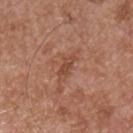Notes:
* follow-up — imaged on a skin check; not biopsied
* diameter — ~3 mm (longest diameter)
* image — 15 mm crop, total-body photography
* automated lesion analysis — an average lesion color of about L≈47 a*≈24 b*≈30 (CIELAB), about 8 CIELAB-L* units darker than the surrounding skin, and a lesion-to-skin contrast of about 6 (normalized; higher = more distinct); peripheral color asymmetry of about 0.5; a classifier nevus-likeness of about 0/100 and a lesion-detection confidence of about 100/100
* body site — the chest
* illumination — white-light illumination
* patient — male, roughly 55 years of age